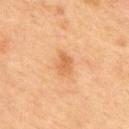No biopsy was performed on this lesion — it was imaged during a full skin examination and was not determined to be concerning.
A male patient, aged 68 to 72.
From the upper back.
The tile uses cross-polarized illumination.
Cropped from a total-body skin-imaging series; the visible field is about 15 mm.